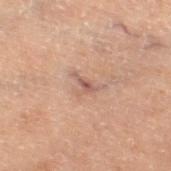workup — catalogued during a skin exam; not biopsied
diameter — ≈3 mm
site — the right thigh
image — ~15 mm crop, total-body skin-cancer survey
illumination — cross-polarized illumination
TBP lesion metrics — a lesion area of about 3.5 mm² and an eccentricity of roughly 0.75; a lesion color around L≈43 a*≈16 b*≈21 in CIELAB, about 7 CIELAB-L* units darker than the surrounding skin, and a normalized lesion–skin contrast near 6; a classifier nevus-likeness of about 0/100 and a detector confidence of about 90 out of 100 that the crop contains a lesion
patient — male, roughly 70 years of age